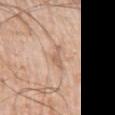Clinical impression:
Captured during whole-body skin photography for melanoma surveillance; the lesion was not biopsied.
Acquisition and patient details:
The patient is a male about 75 years old. The tile uses white-light illumination. The lesion's longest dimension is about 3 mm. Automated tile analysis of the lesion measured a lesion area of about 3 mm², an outline eccentricity of about 0.95 (0 = round, 1 = elongated), and two-axis asymmetry of about 0.6. The software also gave roughly 9 lightness units darker than nearby skin and a lesion-to-skin contrast of about 6 (normalized; higher = more distinct). The software also gave a border-irregularity rating of about 7/10, internal color variation of about 0.5 on a 0–10 scale, and radial color variation of about 0. The software also gave a nevus-likeness score of about 0/100 and a detector confidence of about 85 out of 100 that the crop contains a lesion. From the left upper arm. Cropped from a total-body skin-imaging series; the visible field is about 15 mm.